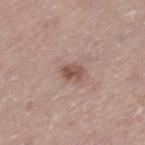lesion size: about 2.5 mm | patient: male, aged 73 to 77 | tile lighting: white-light | automated metrics: about 11 CIELAB-L* units darker than the surrounding skin and a lesion-to-skin contrast of about 8 (normalized; higher = more distinct); a detector confidence of about 100 out of 100 that the crop contains a lesion | body site: the left thigh | acquisition: 15 mm crop, total-body photography.